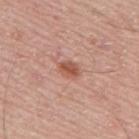Assessment:
The lesion was photographed on a routine skin check and not biopsied; there is no pathology result.
Background:
Automated image analysis of the tile measured a border-irregularity index near 1.5/10, internal color variation of about 2.5 on a 0–10 scale, and a peripheral color-asymmetry measure near 1. It also reported an automated nevus-likeness rating near 90 out of 100 and a detector confidence of about 100 out of 100 that the crop contains a lesion. Cropped from a total-body skin-imaging series; the visible field is about 15 mm. The subject is a male about 75 years old. Imaged with white-light lighting. The lesion's longest dimension is about 2.5 mm. On the mid back.